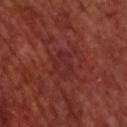Notes:
– workup — total-body-photography surveillance lesion; no biopsy
– patient — male, aged 68–72
– imaging modality — ~15 mm crop, total-body skin-cancer survey
– image-analysis metrics — an average lesion color of about L≈27 a*≈27 b*≈22 (CIELAB), a lesion–skin lightness drop of about 4, and a lesion-to-skin contrast of about 5 (normalized; higher = more distinct)
– diameter — ~4 mm (longest diameter)
– tile lighting — cross-polarized
– anatomic site — the upper back